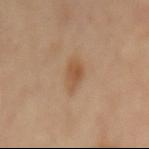{
  "biopsy_status": "not biopsied; imaged during a skin examination",
  "site": "mid back",
  "image": {
    "source": "total-body photography crop",
    "field_of_view_mm": 15
  },
  "lesion_size": {
    "long_diameter_mm_approx": 3.5
  },
  "lighting": "cross-polarized"
}